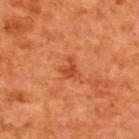Impression:
The lesion was tiled from a total-body skin photograph and was not biopsied.
Image and clinical context:
A close-up tile cropped from a whole-body skin photograph, about 15 mm across. From the upper back. A male subject, about 65 years old. Measured at roughly 2.5 mm in maximum diameter. This is a cross-polarized tile. Automated tile analysis of the lesion measured an average lesion color of about L≈48 a*≈34 b*≈41 (CIELAB), about 9 CIELAB-L* units darker than the surrounding skin, and a normalized border contrast of about 6.5. The analysis additionally found border irregularity of about 4.5 on a 0–10 scale, a color-variation rating of about 1/10, and peripheral color asymmetry of about 0.5. The analysis additionally found a classifier nevus-likeness of about 25/100 and a lesion-detection confidence of about 100/100.